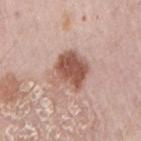{
  "patient": {
    "sex": "male",
    "age_approx": 60
  },
  "image": {
    "source": "total-body photography crop",
    "field_of_view_mm": 15
  },
  "site": "right upper arm"
}